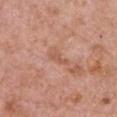Part of a total-body skin-imaging series; this lesion was reviewed on a skin check and was not flagged for biopsy. The lesion is located on the chest. The patient is a female aged approximately 60. A region of skin cropped from a whole-body photographic capture, roughly 15 mm wide. Measured at roughly 3 mm in maximum diameter.